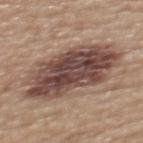biopsy_status: not biopsied; imaged during a skin examination
image:
  source: total-body photography crop
  field_of_view_mm: 15
patient:
  sex: female
  age_approx: 70
site: upper back
automated_metrics:
  area_mm2_approx: 40.0
  eccentricity: 0.85
  shape_asymmetry: 0.2
  cielab_L: 46
  cielab_a: 18
  cielab_b: 22
  vs_skin_darker_L: 16.0
  vs_skin_contrast_norm: 12.0
  border_irregularity_0_10: 3.0
  color_variation_0_10: 7.5
  nevus_likeness_0_100: 50
  lesion_detection_confidence_0_100: 100